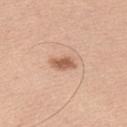Q: Was a biopsy performed?
A: total-body-photography surveillance lesion; no biopsy
Q: Patient demographics?
A: male, approximately 40 years of age
Q: Illumination type?
A: white-light illumination
Q: Automated lesion metrics?
A: a lesion area of about 4.5 mm², an outline eccentricity of about 0.85 (0 = round, 1 = elongated), and a shape-asymmetry score of about 0.3 (0 = symmetric); a border-irregularity index near 3/10, internal color variation of about 2 on a 0–10 scale, and radial color variation of about 0.5
Q: How was this image acquired?
A: 15 mm crop, total-body photography
Q: Lesion location?
A: the back